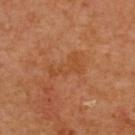Clinical impression:
No biopsy was performed on this lesion — it was imaged during a full skin examination and was not determined to be concerning.
Context:
The lesion is on the upper back. About 4 mm across. A female patient, aged 38–42. This is a cross-polarized tile. A region of skin cropped from a whole-body photographic capture, roughly 15 mm wide.